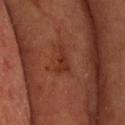Q: Is there a histopathology result?
A: total-body-photography surveillance lesion; no biopsy
Q: What are the patient's age and sex?
A: male, approximately 65 years of age
Q: Illumination type?
A: cross-polarized illumination
Q: Automated lesion metrics?
A: a border-irregularity index near 5.5/10, a color-variation rating of about 1.5/10, and radial color variation of about 0.5
Q: How was this image acquired?
A: total-body-photography crop, ~15 mm field of view
Q: What is the anatomic site?
A: the back